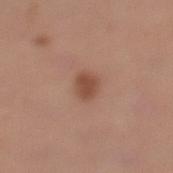Q: Was this lesion biopsied?
A: imaged on a skin check; not biopsied
Q: Where on the body is the lesion?
A: the left lower leg
Q: What did automated image analysis measure?
A: a color-variation rating of about 1.5/10
Q: Illumination type?
A: white-light illumination
Q: What are the patient's age and sex?
A: female, in their mid-30s
Q: How large is the lesion?
A: ≈2.5 mm
Q: How was this image acquired?
A: total-body-photography crop, ~15 mm field of view